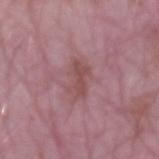Clinical impression:
Imaged during a routine full-body skin examination; the lesion was not biopsied and no histopathology is available.
Image and clinical context:
About 4 mm across. Imaged with white-light lighting. The lesion is located on the right upper arm. A 15 mm close-up extracted from a 3D total-body photography capture. Automated tile analysis of the lesion measured a lesion area of about 5.5 mm², a shape eccentricity near 0.9, and two-axis asymmetry of about 0.35. The analysis additionally found a lesion–skin lightness drop of about 7 and a normalized lesion–skin contrast near 6.5. The analysis additionally found a border-irregularity rating of about 4.5/10, a color-variation rating of about 1.5/10, and peripheral color asymmetry of about 0.5. The subject is a male approximately 65 years of age.